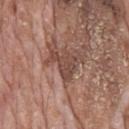No biopsy was performed on this lesion — it was imaged during a full skin examination and was not determined to be concerning.
From the mid back.
A roughly 15 mm field-of-view crop from a total-body skin photograph.
A male patient, aged around 70.